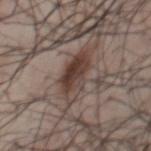Q: Was this lesion biopsied?
A: imaged on a skin check; not biopsied
Q: Patient demographics?
A: male, about 55 years old
Q: What is the imaging modality?
A: 15 mm crop, total-body photography
Q: What is the lesion's diameter?
A: ≈5.5 mm
Q: Where on the body is the lesion?
A: the chest
Q: Illumination type?
A: white-light